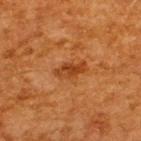Notes:
* notes: total-body-photography surveillance lesion; no biopsy
* site: the back
* tile lighting: cross-polarized illumination
* lesion diameter: ~4 mm (longest diameter)
* patient: male, about 65 years old
* image: total-body-photography crop, ~15 mm field of view